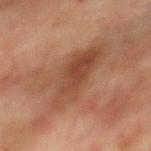Longest diameter approximately 7.5 mm.
A male patient, aged 73–77.
Captured under cross-polarized illumination.
The lesion-visualizer software estimated a lesion area of about 20 mm², an eccentricity of roughly 0.85, and two-axis asymmetry of about 0.35. The analysis additionally found a border-irregularity rating of about 5/10 and a within-lesion color-variation index near 4.5/10. And it measured a nevus-likeness score of about 0/100.
Cropped from a whole-body photographic skin survey; the tile spans about 15 mm.
Located on the mid back.